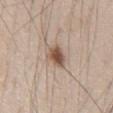<case>
  <biopsy_status>not biopsied; imaged during a skin examination</biopsy_status>
  <automated_metrics>
    <lesion_detection_confidence_0_100>100</lesion_detection_confidence_0_100>
  </automated_metrics>
  <site>abdomen</site>
  <image>
    <source>total-body photography crop</source>
    <field_of_view_mm>15</field_of_view_mm>
  </image>
  <patient>
    <sex>male</sex>
    <age_approx>45</age_approx>
  </patient>
</case>